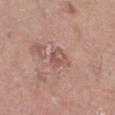Context: Approximately 2.5 mm at its widest. The lesion is located on the left lower leg. A female patient in their mid- to late 60s. Cropped from a whole-body photographic skin survey; the tile spans about 15 mm.